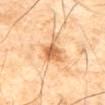Imaged during a routine full-body skin examination; the lesion was not biopsied and no histopathology is available. Cropped from a whole-body photographic skin survey; the tile spans about 15 mm. On the abdomen. A male subject, aged around 65. Longest diameter approximately 4 mm. This is a cross-polarized tile. Automated tile analysis of the lesion measured a lesion area of about 7 mm², an outline eccentricity of about 0.8 (0 = round, 1 = elongated), and two-axis asymmetry of about 0.35. And it measured a lesion-detection confidence of about 100/100.